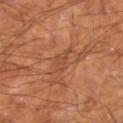Automated image analysis of the tile measured a lesion color around L≈46 a*≈24 b*≈32 in CIELAB and a normalized lesion–skin contrast near 4.5. It also reported a within-lesion color-variation index near 1.5/10. It also reported a classifier nevus-likeness of about 0/100 and a lesion-detection confidence of about 70/100.
Imaged with cross-polarized lighting.
Measured at roughly 3 mm in maximum diameter.
A male patient aged 58 to 62.
Located on the leg.
A region of skin cropped from a whole-body photographic capture, roughly 15 mm wide.